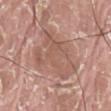biopsy status=total-body-photography surveillance lesion; no biopsy
subject=male, about 40 years old
image=~15 mm crop, total-body skin-cancer survey
lesion diameter=≈7 mm
TBP lesion metrics=a nevus-likeness score of about 0/100 and lesion-presence confidence of about 70/100
site=the left thigh